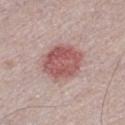This lesion was catalogued during total-body skin photography and was not selected for biopsy. A region of skin cropped from a whole-body photographic capture, roughly 15 mm wide. The patient is a male aged 28–32.